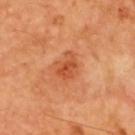{"biopsy_status": "not biopsied; imaged during a skin examination", "site": "upper back", "automated_metrics": {"area_mm2_approx": 6.5, "eccentricity": 0.6, "shape_asymmetry": 0.35, "border_irregularity_0_10": 3.5, "color_variation_0_10": 3.5, "peripheral_color_asymmetry": 1.5, "nevus_likeness_0_100": 90, "lesion_detection_confidence_0_100": 100}, "image": {"source": "total-body photography crop", "field_of_view_mm": 15}, "lighting": "cross-polarized", "patient": {"sex": "male", "age_approx": 65}}